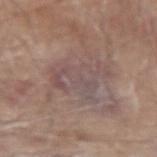<tbp_lesion>
<lighting>white-light</lighting>
<site>arm</site>
<image>
  <source>total-body photography crop</source>
  <field_of_view_mm>15</field_of_view_mm>
</image>
<lesion_size>
  <long_diameter_mm_approx>5.5</long_diameter_mm_approx>
</lesion_size>
<patient>
  <sex>male</sex>
  <age_approx>65</age_approx>
</patient>
<automated_metrics>
  <lesion_detection_confidence_0_100>70</lesion_detection_confidence_0_100>
</automated_metrics>
</tbp_lesion>